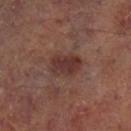{"biopsy_status": "not biopsied; imaged during a skin examination", "site": "leg", "image": {"source": "total-body photography crop", "field_of_view_mm": 15}, "patient": {"sex": "male", "age_approx": 65}, "lesion_size": {"long_diameter_mm_approx": 4.0}, "automated_metrics": {"eccentricity": 0.75, "shape_asymmetry": 0.15, "border_irregularity_0_10": 1.5, "peripheral_color_asymmetry": 1.0, "nevus_likeness_0_100": 65, "lesion_detection_confidence_0_100": 100}, "lighting": "cross-polarized"}